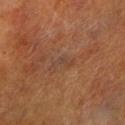Assessment: The lesion was tiled from a total-body skin photograph and was not biopsied. Clinical summary: Located on the leg. A male patient, in their 70s. Automated tile analysis of the lesion measured a border-irregularity index near 6.5/10 and a within-lesion color-variation index near 0/10. The software also gave a nevus-likeness score of about 0/100 and a detector confidence of about 90 out of 100 that the crop contains a lesion. A 15 mm crop from a total-body photograph taken for skin-cancer surveillance. About 3 mm across.